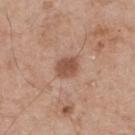Imaged during a routine full-body skin examination; the lesion was not biopsied and no histopathology is available.
A close-up tile cropped from a whole-body skin photograph, about 15 mm across.
A male subject, approximately 55 years of age.
The lesion-visualizer software estimated a footprint of about 5 mm².
About 2.5 mm across.
Captured under white-light illumination.
The lesion is on the back.